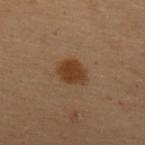workup=no biopsy performed (imaged during a skin exam) | acquisition=15 mm crop, total-body photography | illumination=cross-polarized | subject=female, in their 50s | image-analysis metrics=a border-irregularity rating of about 1.5/10, a within-lesion color-variation index near 2/10, and radial color variation of about 1 | anatomic site=the upper back | lesion diameter=~3.5 mm (longest diameter).